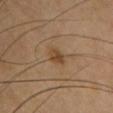Notes:
- biopsy status · no biopsy performed (imaged during a skin exam)
- patient · female, in their mid- to late 40s
- image-analysis metrics · a lesion color around L≈45 a*≈17 b*≈33 in CIELAB and a lesion–skin lightness drop of about 9; a classifier nevus-likeness of about 30/100 and a detector confidence of about 100 out of 100 that the crop contains a lesion
- image · ~15 mm crop, total-body skin-cancer survey
- size · ≈2.5 mm
- anatomic site · the chest
- illumination · cross-polarized illumination